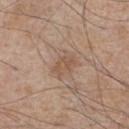Captured during whole-body skin photography for melanoma surveillance; the lesion was not biopsied.
A 15 mm close-up extracted from a 3D total-body photography capture.
The subject is a male aged approximately 55.
The lesion is on the chest.
Longest diameter approximately 3 mm.
The tile uses white-light illumination.